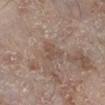follow-up: catalogued during a skin exam; not biopsied
diameter: ≈2.5 mm
location: the right lower leg
illumination: white-light illumination
patient: male, in their mid- to late 60s
image source: total-body-photography crop, ~15 mm field of view